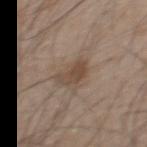Assessment:
Recorded during total-body skin imaging; not selected for excision or biopsy.
Context:
The lesion's longest dimension is about 3 mm. The tile uses white-light illumination. The lesion-visualizer software estimated an automated nevus-likeness rating near 55 out of 100 and lesion-presence confidence of about 100/100. From the mid back. A male subject, aged approximately 50. Cropped from a total-body skin-imaging series; the visible field is about 15 mm.